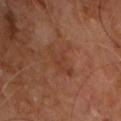{
  "biopsy_status": "not biopsied; imaged during a skin examination",
  "automated_metrics": {
    "area_mm2_approx": 6.0,
    "eccentricity": 0.8,
    "shape_asymmetry": 0.4,
    "cielab_L": 37,
    "cielab_a": 22,
    "cielab_b": 29,
    "border_irregularity_0_10": 5.5,
    "nevus_likeness_0_100": 0,
    "lesion_detection_confidence_0_100": 100
  },
  "patient": {
    "sex": "male",
    "age_approx": 60
  },
  "lighting": "cross-polarized",
  "lesion_size": {
    "long_diameter_mm_approx": 4.0
  },
  "image": {
    "source": "total-body photography crop",
    "field_of_view_mm": 15
  },
  "site": "upper back"
}